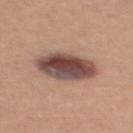Assessment:
Recorded during total-body skin imaging; not selected for excision or biopsy.
Background:
Measured at roughly 6 mm in maximum diameter. A 15 mm crop from a total-body photograph taken for skin-cancer surveillance. Located on the chest. The lesion-visualizer software estimated a lesion area of about 17 mm² and a symmetry-axis asymmetry near 0.1. It also reported roughly 18 lightness units darker than nearby skin and a normalized border contrast of about 13. It also reported border irregularity of about 1.5 on a 0–10 scale, a color-variation rating of about 7.5/10, and radial color variation of about 2. And it measured an automated nevus-likeness rating near 15 out of 100. A female patient, approximately 35 years of age.